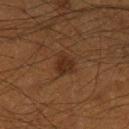follow-up=no biopsy performed (imaged during a skin exam); lighting=cross-polarized illumination; image source=~15 mm crop, total-body skin-cancer survey; location=the leg; lesion diameter=~3 mm (longest diameter); subject=male, aged 58 to 62.